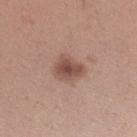Recorded during total-body skin imaging; not selected for excision or biopsy.
Measured at roughly 3.5 mm in maximum diameter.
Captured under white-light illumination.
The lesion-visualizer software estimated an area of roughly 8 mm², a shape eccentricity near 0.6, and a symmetry-axis asymmetry near 0.25. And it measured an automated nevus-likeness rating near 85 out of 100.
The lesion is on the left forearm.
The patient is a female aged around 30.
A 15 mm close-up tile from a total-body photography series done for melanoma screening.